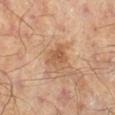{"biopsy_status": "not biopsied; imaged during a skin examination", "lighting": "cross-polarized", "lesion_size": {"long_diameter_mm_approx": 3.5}, "image": {"source": "total-body photography crop", "field_of_view_mm": 15}, "patient": {"sex": "male", "age_approx": 70}, "site": "right lower leg"}